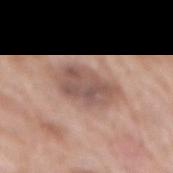Located on the mid back.
A female subject aged 73 to 77.
Imaged with white-light lighting.
A roughly 15 mm field-of-view crop from a total-body skin photograph.